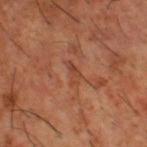Part of a total-body skin-imaging series; this lesion was reviewed on a skin check and was not flagged for biopsy.
A lesion tile, about 15 mm wide, cut from a 3D total-body photograph.
Measured at roughly 2.5 mm in maximum diameter.
Automated image analysis of the tile measured a mean CIELAB color near L≈44 a*≈25 b*≈33, about 7 CIELAB-L* units darker than the surrounding skin, and a normalized border contrast of about 5.5. It also reported border irregularity of about 5 on a 0–10 scale, a color-variation rating of about 0/10, and peripheral color asymmetry of about 0. The analysis additionally found a detector confidence of about 65 out of 100 that the crop contains a lesion.
Captured under cross-polarized illumination.
On the right thigh.
The patient is a male roughly 65 years of age.